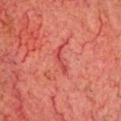biopsy_status: not biopsied; imaged during a skin examination
lighting: cross-polarized
site: head or neck
image:
  source: total-body photography crop
  field_of_view_mm: 15
automated_metrics:
  border_irregularity_0_10: 4.5
  color_variation_0_10: 0.0
  nevus_likeness_0_100: 0
  lesion_detection_confidence_0_100: 65
patient:
  sex: male
  age_approx: 60
lesion_size:
  long_diameter_mm_approx: 3.5